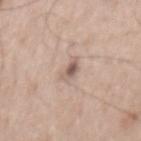* follow-up: no biopsy performed (imaged during a skin exam)
* tile lighting: white-light illumination
* lesion size: ≈2.5 mm
* body site: the back
* imaging modality: total-body-photography crop, ~15 mm field of view
* automated lesion analysis: an area of roughly 3.5 mm², a shape eccentricity near 0.75, and a symmetry-axis asymmetry near 0.35; a border-irregularity rating of about 3/10, a within-lesion color-variation index near 5/10, and peripheral color asymmetry of about 1.5
* patient: male, aged approximately 50